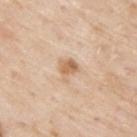No biopsy was performed on this lesion — it was imaged during a full skin examination and was not determined to be concerning.
A male patient about 80 years old.
A 15 mm close-up tile from a total-body photography series done for melanoma screening.
The lesion is located on the upper back.
Imaged with white-light lighting.